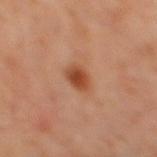{
  "biopsy_status": "not biopsied; imaged during a skin examination",
  "lesion_size": {
    "long_diameter_mm_approx": 3.0
  },
  "image": {
    "source": "total-body photography crop",
    "field_of_view_mm": 15
  },
  "patient": {
    "sex": "male",
    "age_approx": 60
  },
  "site": "mid back"
}